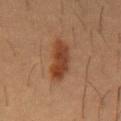Captured during whole-body skin photography for melanoma surveillance; the lesion was not biopsied. The lesion-visualizer software estimated an eccentricity of roughly 0.95 and a symmetry-axis asymmetry near 0.25. It also reported a border-irregularity index near 3.5/10 and a within-lesion color-variation index near 2.5/10. Imaged with cross-polarized lighting. A male patient aged approximately 55. The lesion's longest dimension is about 6 mm. The lesion is located on the mid back. A 15 mm close-up tile from a total-body photography series done for melanoma screening.